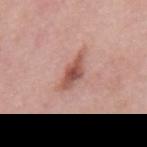biopsy status: total-body-photography surveillance lesion; no biopsy | imaging modality: total-body-photography crop, ~15 mm field of view | tile lighting: white-light illumination | site: the mid back | TBP lesion metrics: internal color variation of about 4.5 on a 0–10 scale | patient: male, about 55 years old | lesion size: about 5 mm.